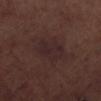The lesion was photographed on a routine skin check and not biopsied; there is no pathology result. The lesion-visualizer software estimated a lesion area of about 13 mm². The software also gave a mean CIELAB color near L≈25 a*≈16 b*≈15, about 4 CIELAB-L* units darker than the surrounding skin, and a lesion-to-skin contrast of about 6 (normalized; higher = more distinct). And it measured border irregularity of about 2.5 on a 0–10 scale and peripheral color asymmetry of about 0.5. A 15 mm close-up tile from a total-body photography series done for melanoma screening. About 4.5 mm across. Located on the left lower leg. The patient is a male aged 68–72.